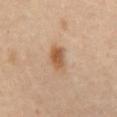Q: Was a biopsy performed?
A: total-body-photography surveillance lesion; no biopsy
Q: Who is the patient?
A: female, aged 53–57
Q: What is the imaging modality?
A: ~15 mm tile from a whole-body skin photo
Q: Automated lesion metrics?
A: a mean CIELAB color near L≈58 a*≈20 b*≈36, roughly 11 lightness units darker than nearby skin, and a normalized lesion–skin contrast near 8.5
Q: How was the tile lit?
A: cross-polarized
Q: Where on the body is the lesion?
A: the abdomen